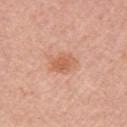workup = catalogued during a skin exam; not biopsied.